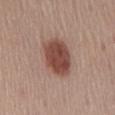notes: no biopsy performed (imaged during a skin exam) | acquisition: 15 mm crop, total-body photography | location: the abdomen | lesion diameter: about 5 mm | image-analysis metrics: about 14 CIELAB-L* units darker than the surrounding skin and a normalized lesion–skin contrast near 10.5; a nevus-likeness score of about 100/100 and lesion-presence confidence of about 100/100 | subject: male, aged around 55.